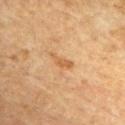| key | value |
|---|---|
| biopsy status | total-body-photography surveillance lesion; no biopsy |
| anatomic site | the chest |
| TBP lesion metrics | a lesion area of about 3 mm², an outline eccentricity of about 0.9 (0 = round, 1 = elongated), and a symmetry-axis asymmetry near 0.4; a mean CIELAB color near L≈54 a*≈21 b*≈38, about 8 CIELAB-L* units darker than the surrounding skin, and a lesion-to-skin contrast of about 6.5 (normalized; higher = more distinct) |
| tile lighting | cross-polarized |
| subject | male, about 65 years old |
| acquisition | ~15 mm crop, total-body skin-cancer survey |